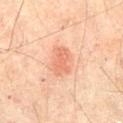| key | value |
|---|---|
| follow-up | no biopsy performed (imaged during a skin exam) |
| location | the abdomen |
| lighting | cross-polarized |
| diameter | about 4 mm |
| patient | male, aged approximately 65 |
| image source | 15 mm crop, total-body photography |